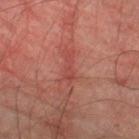Assessment:
The lesion was tiled from a total-body skin photograph and was not biopsied.
Context:
Approximately 3 mm at its widest. Imaged with cross-polarized lighting. A male subject, aged 73–77. From the right thigh. A lesion tile, about 15 mm wide, cut from a 3D total-body photograph.